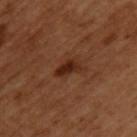Approximately 3 mm at its widest.
This is a cross-polarized tile.
Located on the upper back.
The patient is a male aged approximately 50.
A 15 mm close-up tile from a total-body photography series done for melanoma screening.